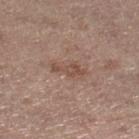Imaged during a routine full-body skin examination; the lesion was not biopsied and no histopathology is available. The tile uses white-light illumination. Located on the leg. A region of skin cropped from a whole-body photographic capture, roughly 15 mm wide. A female subject, approximately 65 years of age.